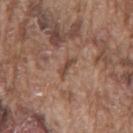workup — catalogued during a skin exam; not biopsied | tile lighting — white-light illumination | patient — male, aged approximately 75 | TBP lesion metrics — an area of roughly 3 mm², a shape eccentricity near 0.95, and two-axis asymmetry of about 0.3; a lesion color around L≈46 a*≈19 b*≈26 in CIELAB, about 9 CIELAB-L* units darker than the surrounding skin, and a lesion-to-skin contrast of about 7 (normalized; higher = more distinct) | anatomic site — the back | acquisition — total-body-photography crop, ~15 mm field of view.